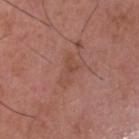Assessment: Part of a total-body skin-imaging series; this lesion was reviewed on a skin check and was not flagged for biopsy. Background: A 15 mm crop from a total-body photograph taken for skin-cancer surveillance. Automated tile analysis of the lesion measured a lesion color around L≈48 a*≈23 b*≈28 in CIELAB, about 6 CIELAB-L* units darker than the surrounding skin, and a normalized border contrast of about 5. The software also gave internal color variation of about 1.5 on a 0–10 scale and peripheral color asymmetry of about 0.5. And it measured a nevus-likeness score of about 0/100 and lesion-presence confidence of about 100/100. The recorded lesion diameter is about 3.5 mm. Imaged with white-light lighting. Located on the head or neck. A male patient, aged 48–52.